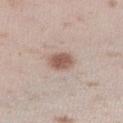No biopsy was performed on this lesion — it was imaged during a full skin examination and was not determined to be concerning. On the right lower leg. A 15 mm crop from a total-body photograph taken for skin-cancer surveillance. This is a white-light tile. The subject is a female approximately 25 years of age. The lesion's longest dimension is about 3 mm.